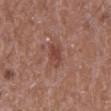| key | value |
|---|---|
| biopsy status | total-body-photography surveillance lesion; no biopsy |
| automated metrics | an area of roughly 5.5 mm², an eccentricity of roughly 0.65, and a symmetry-axis asymmetry near 0.2; an average lesion color of about L≈45 a*≈23 b*≈26 (CIELAB), a lesion–skin lightness drop of about 8, and a normalized lesion–skin contrast near 6 |
| patient | male, roughly 50 years of age |
| location | the leg |
| tile lighting | white-light |
| lesion diameter | about 2.5 mm |
| imaging modality | total-body-photography crop, ~15 mm field of view |